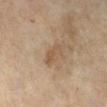The lesion was tiled from a total-body skin photograph and was not biopsied.
A female subject about 70 years old.
The recorded lesion diameter is about 3.5 mm.
Captured under cross-polarized illumination.
A roughly 15 mm field-of-view crop from a total-body skin photograph.
The lesion is located on the leg.
The lesion-visualizer software estimated a footprint of about 4.5 mm², an outline eccentricity of about 0.9 (0 = round, 1 = elongated), and two-axis asymmetry of about 0.4. And it measured a within-lesion color-variation index near 2/10 and a peripheral color-asymmetry measure near 0.5. It also reported a nevus-likeness score of about 5/100 and lesion-presence confidence of about 100/100.